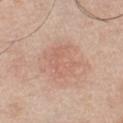Recorded during total-body skin imaging; not selected for excision or biopsy.